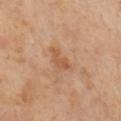Q: What is the lesion's diameter?
A: about 4 mm
Q: Patient demographics?
A: male, about 65 years old
Q: How was the tile lit?
A: cross-polarized illumination
Q: How was this image acquired?
A: total-body-photography crop, ~15 mm field of view